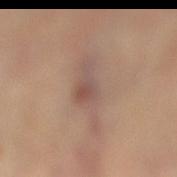Part of a total-body skin-imaging series; this lesion was reviewed on a skin check and was not flagged for biopsy.
This is a cross-polarized tile.
The patient is a female aged approximately 65.
Automated image analysis of the tile measured a border-irregularity rating of about 5/10 and radial color variation of about 1.5.
Measured at roughly 7 mm in maximum diameter.
Cropped from a whole-body photographic skin survey; the tile spans about 15 mm.
Located on the right lower leg.